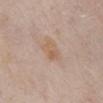<record>
  <biopsy_status>not biopsied; imaged during a skin examination</biopsy_status>
  <patient>
    <sex>male</sex>
    <age_approx>85+</age_approx>
  </patient>
  <image>
    <source>total-body photography crop</source>
    <field_of_view_mm>15</field_of_view_mm>
  </image>
  <lighting>white-light</lighting>
  <site>chest</site>
  <lesion_size>
    <long_diameter_mm_approx>3.0</long_diameter_mm_approx>
  </lesion_size>
</record>